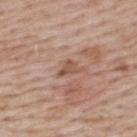The lesion was tiled from a total-body skin photograph and was not biopsied. On the upper back. The lesion-visualizer software estimated a within-lesion color-variation index near 1/10. The software also gave a classifier nevus-likeness of about 0/100 and lesion-presence confidence of about 100/100. The subject is a male aged approximately 65. Cropped from a whole-body photographic skin survey; the tile spans about 15 mm. Imaged with white-light lighting.